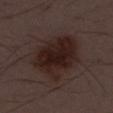Impression:
Imaged during a routine full-body skin examination; the lesion was not biopsied and no histopathology is available.
Image and clinical context:
Automated image analysis of the tile measured internal color variation of about 3.5 on a 0–10 scale and radial color variation of about 1. The patient is a male aged approximately 55. A roughly 15 mm field-of-view crop from a total-body skin photograph. The recorded lesion diameter is about 6 mm. The lesion is on the abdomen.